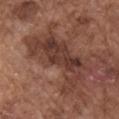Q: Is there a histopathology result?
A: no biopsy performed (imaged during a skin exam)
Q: How was this image acquired?
A: 15 mm crop, total-body photography
Q: What is the lesion's diameter?
A: ≈9 mm
Q: What are the patient's age and sex?
A: male, approximately 75 years of age
Q: Lesion location?
A: the right upper arm